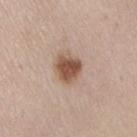biopsy_status: not biopsied; imaged during a skin examination
image:
  source: total-body photography crop
  field_of_view_mm: 15
patient:
  sex: male
  age_approx: 50
site: lower back
lesion_size:
  long_diameter_mm_approx: 3.5